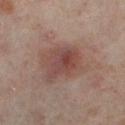<tbp_lesion>
<biopsy_status>not biopsied; imaged during a skin examination</biopsy_status>
<automated_metrics>
  <color_variation_0_10>5.5</color_variation_0_10>
  <peripheral_color_asymmetry>1.5</peripheral_color_asymmetry>
  <nevus_likeness_0_100>30</nevus_likeness_0_100>
</automated_metrics>
<patient>
  <sex>female</sex>
  <age_approx>50</age_approx>
</patient>
<site>leg</site>
<image>
  <source>total-body photography crop</source>
  <field_of_view_mm>15</field_of_view_mm>
</image>
<lesion_size>
  <long_diameter_mm_approx>4.5</long_diameter_mm_approx>
</lesion_size>
<lighting>cross-polarized</lighting>
</tbp_lesion>